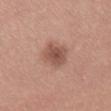{"biopsy_status": "not biopsied; imaged during a skin examination", "image": {"source": "total-body photography crop", "field_of_view_mm": 15}, "lighting": "white-light", "patient": {"sex": "female", "age_approx": 40}, "site": "right thigh"}